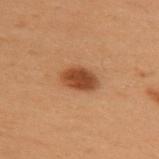follow-up: imaged on a skin check; not biopsied
patient: female, about 50 years old
TBP lesion metrics: about 12 CIELAB-L* units darker than the surrounding skin and a normalized lesion–skin contrast near 10
imaging modality: total-body-photography crop, ~15 mm field of view
lighting: cross-polarized illumination
site: the upper back